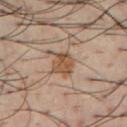Q: What lighting was used for the tile?
A: cross-polarized illumination
Q: What is the imaging modality?
A: ~15 mm crop, total-body skin-cancer survey
Q: Automated lesion metrics?
A: a lesion–skin lightness drop of about 10 and a normalized border contrast of about 8.5; a border-irregularity index near 2/10, internal color variation of about 3.5 on a 0–10 scale, and radial color variation of about 1.5
Q: Lesion location?
A: the chest
Q: Who is the patient?
A: male, aged 38 to 42